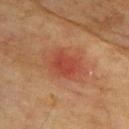Assessment:
Captured during whole-body skin photography for melanoma surveillance; the lesion was not biopsied.
Acquisition and patient details:
On the upper back. A 15 mm crop from a total-body photograph taken for skin-cancer surveillance. The tile uses cross-polarized illumination. Automated image analysis of the tile measured a lesion area of about 8.5 mm², a shape eccentricity near 0.6, and a shape-asymmetry score of about 0.2 (0 = symmetric). And it measured an average lesion color of about L≈39 a*≈28 b*≈29 (CIELAB), roughly 7 lightness units darker than nearby skin, and a normalized lesion–skin contrast near 6.5. It also reported a border-irregularity index near 2/10, internal color variation of about 2 on a 0–10 scale, and radial color variation of about 0.5. A male patient, in their mid-70s. Longest diameter approximately 3.5 mm.